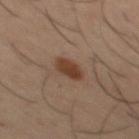– notes · catalogued during a skin exam; not biopsied